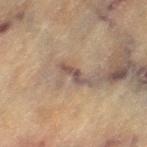Q: Is there a histopathology result?
A: no biopsy performed (imaged during a skin exam)
Q: What did automated image analysis measure?
A: a lesion area of about 2.5 mm², a shape eccentricity near 0.95, and a symmetry-axis asymmetry near 0.5; a border-irregularity index near 6/10 and a within-lesion color-variation index near 0/10
Q: What are the patient's age and sex?
A: female, approximately 80 years of age
Q: Illumination type?
A: cross-polarized illumination
Q: What kind of image is this?
A: ~15 mm crop, total-body skin-cancer survey
Q: What is the anatomic site?
A: the leg
Q: How large is the lesion?
A: about 3 mm